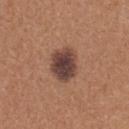Impression:
This lesion was catalogued during total-body skin photography and was not selected for biopsy.
Background:
The lesion is located on the upper back. A close-up tile cropped from a whole-body skin photograph, about 15 mm across. The patient is a female approximately 40 years of age.